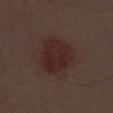Clinical summary: The recorded lesion diameter is about 5 mm. From the abdomen. The tile uses white-light illumination. A male patient in their mid-50s. A lesion tile, about 15 mm wide, cut from a 3D total-body photograph.